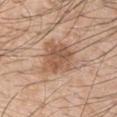<record>
<biopsy_status>not biopsied; imaged during a skin examination</biopsy_status>
<patient>
  <sex>male</sex>
  <age_approx>50</age_approx>
</patient>
<lighting>white-light</lighting>
<site>left upper arm</site>
<image>
  <source>total-body photography crop</source>
  <field_of_view_mm>15</field_of_view_mm>
</image>
<automated_metrics>
  <cielab_L>55</cielab_L>
  <cielab_a>20</cielab_a>
  <cielab_b>31</cielab_b>
  <vs_skin_darker_L>10.0</vs_skin_darker_L>
  <border_irregularity_0_10>2.5</border_irregularity_0_10>
  <peripheral_color_asymmetry>1.5</peripheral_color_asymmetry>
  <nevus_likeness_0_100>70</nevus_likeness_0_100>
  <lesion_detection_confidence_0_100>100</lesion_detection_confidence_0_100>
</automated_metrics>
</record>